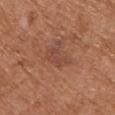Imaged during a routine full-body skin examination; the lesion was not biopsied and no histopathology is available.